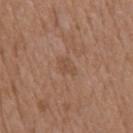workup=imaged on a skin check; not biopsied | lesion size=about 2.5 mm | subject=male, in their mid-70s | anatomic site=the chest | tile lighting=white-light | imaging modality=~15 mm tile from a whole-body skin photo.